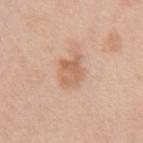Clinical impression: No biopsy was performed on this lesion — it was imaged during a full skin examination and was not determined to be concerning.